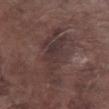follow-up = imaged on a skin check; not biopsied
image source = ~15 mm crop, total-body skin-cancer survey
TBP lesion metrics = a lesion area of about 19 mm², a shape eccentricity near 0.9, and two-axis asymmetry of about 0.45; border irregularity of about 8.5 on a 0–10 scale and radial color variation of about 1; a nevus-likeness score of about 0/100 and lesion-presence confidence of about 55/100
site = the right lower leg
lesion size = ~8 mm (longest diameter)
subject = male, aged around 75
illumination = white-light